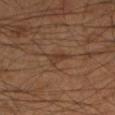Imaged during a routine full-body skin examination; the lesion was not biopsied and no histopathology is available.
Cropped from a whole-body photographic skin survey; the tile spans about 15 mm.
The patient is a male aged around 55.
The lesion is located on the leg.